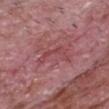Assessment:
This lesion was catalogued during total-body skin photography and was not selected for biopsy.
Image and clinical context:
The lesion-visualizer software estimated an area of roughly 5 mm², an eccentricity of roughly 0.85, and a shape-asymmetry score of about 0.75 (0 = symmetric). It also reported a border-irregularity index near 9.5/10 and a peripheral color-asymmetry measure near 0. The subject is a male in their mid- to late 60s. The lesion is located on the head or neck. A lesion tile, about 15 mm wide, cut from a 3D total-body photograph. Measured at roughly 4 mm in maximum diameter.